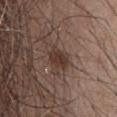| field | value |
|---|---|
| workup | catalogued during a skin exam; not biopsied |
| location | the front of the torso |
| acquisition | 15 mm crop, total-body photography |
| subject | male, approximately 55 years of age |